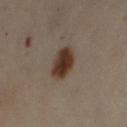body site = the left lower leg
image source = ~15 mm tile from a whole-body skin photo
subject = female, roughly 50 years of age
diameter = ~3.5 mm (longest diameter)